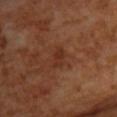biopsy status: total-body-photography surveillance lesion; no biopsy | anatomic site: the back | automated lesion analysis: an area of roughly 3.5 mm², an eccentricity of roughly 0.85, and two-axis asymmetry of about 0.4; roughly 6 lightness units darker than nearby skin and a lesion-to-skin contrast of about 6 (normalized; higher = more distinct); an automated nevus-likeness rating near 0 out of 100 and lesion-presence confidence of about 100/100 | lighting: cross-polarized | size: ≈2.5 mm | acquisition: ~15 mm crop, total-body skin-cancer survey | patient: female.